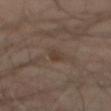Q: Was a biopsy performed?
A: imaged on a skin check; not biopsied
Q: Automated lesion metrics?
A: a lesion color around L≈36 a*≈14 b*≈22 in CIELAB, roughly 7 lightness units darker than nearby skin, and a normalized border contrast of about 6.5; border irregularity of about 3.5 on a 0–10 scale, a within-lesion color-variation index near 0/10, and peripheral color asymmetry of about 0; a nevus-likeness score of about 0/100 and a detector confidence of about 90 out of 100 that the crop contains a lesion
Q: What is the imaging modality?
A: ~15 mm crop, total-body skin-cancer survey
Q: Illumination type?
A: cross-polarized
Q: What are the patient's age and sex?
A: male, roughly 50 years of age
Q: Lesion location?
A: the mid back